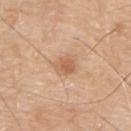<case>
<biopsy_status>not biopsied; imaged during a skin examination</biopsy_status>
<site>upper back</site>
<patient>
  <sex>male</sex>
  <age_approx>80</age_approx>
</patient>
<lighting>white-light</lighting>
<image>
  <source>total-body photography crop</source>
  <field_of_view_mm>15</field_of_view_mm>
</image>
<lesion_size>
  <long_diameter_mm_approx>3.0</long_diameter_mm_approx>
</lesion_size>
</case>